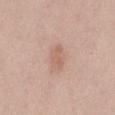Clinical impression:
No biopsy was performed on this lesion — it was imaged during a full skin examination and was not determined to be concerning.
Clinical summary:
The patient is a female aged 38–42. The total-body-photography lesion software estimated a footprint of about 5 mm², an outline eccentricity of about 0.75 (0 = round, 1 = elongated), and a symmetry-axis asymmetry near 0.25. It also reported a border-irregularity index near 2.5/10, a color-variation rating of about 1.5/10, and a peripheral color-asymmetry measure near 0.5. It also reported a nevus-likeness score of about 25/100 and a lesion-detection confidence of about 100/100. About 3 mm across. Cropped from a whole-body photographic skin survey; the tile spans about 15 mm. From the left thigh.